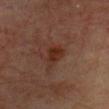Clinical impression: No biopsy was performed on this lesion — it was imaged during a full skin examination and was not determined to be concerning. Context: The tile uses cross-polarized illumination. Automated tile analysis of the lesion measured an average lesion color of about L≈26 a*≈19 b*≈24 (CIELAB), about 7 CIELAB-L* units darker than the surrounding skin, and a lesion-to-skin contrast of about 8.5 (normalized; higher = more distinct). It also reported border irregularity of about 3 on a 0–10 scale and internal color variation of about 2.5 on a 0–10 scale. From the upper back. A close-up tile cropped from a whole-body skin photograph, about 15 mm across. A male patient in their 60s. Approximately 2.5 mm at its widest.